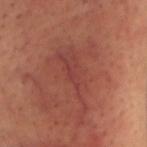| field | value |
|---|---|
| biopsy status | imaged on a skin check; not biopsied |
| body site | the head or neck |
| lesion size | about 9 mm |
| subject | male, roughly 45 years of age |
| image source | ~15 mm crop, total-body skin-cancer survey |
| illumination | cross-polarized |